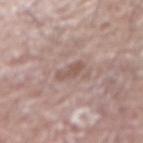Impression:
Imaged during a routine full-body skin examination; the lesion was not biopsied and no histopathology is available.
Context:
A male subject in their mid-70s. Measured at roughly 3 mm in maximum diameter. The lesion-visualizer software estimated a mean CIELAB color near L≈54 a*≈18 b*≈23, about 9 CIELAB-L* units darker than the surrounding skin, and a lesion-to-skin contrast of about 6 (normalized; higher = more distinct). It also reported a border-irregularity rating of about 4.5/10, internal color variation of about 0.5 on a 0–10 scale, and a peripheral color-asymmetry measure near 0. Located on the right upper arm. The tile uses white-light illumination. Cropped from a total-body skin-imaging series; the visible field is about 15 mm.